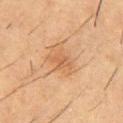- workup · catalogued during a skin exam; not biopsied
- patient · male, approximately 55 years of age
- imaging modality · ~15 mm tile from a whole-body skin photo
- site · the upper back
- lighting · cross-polarized illumination